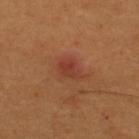The lesion was photographed on a routine skin check and not biopsied; there is no pathology result.
Measured at roughly 3.5 mm in maximum diameter.
The lesion is located on the upper back.
A male subject, aged approximately 65.
This image is a 15 mm lesion crop taken from a total-body photograph.
Imaged with cross-polarized lighting.